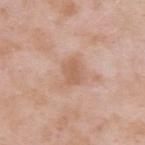Part of a total-body skin-imaging series; this lesion was reviewed on a skin check and was not flagged for biopsy. A male patient, approximately 55 years of age. From the upper back. A close-up tile cropped from a whole-body skin photograph, about 15 mm across.